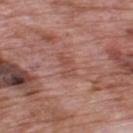Imaged during a routine full-body skin examination; the lesion was not biopsied and no histopathology is available. An algorithmic analysis of the crop reported border irregularity of about 5 on a 0–10 scale, a within-lesion color-variation index near 2/10, and a peripheral color-asymmetry measure near 0.5. Measured at roughly 3.5 mm in maximum diameter. A roughly 15 mm field-of-view crop from a total-body skin photograph. Captured under white-light illumination. A male patient, aged approximately 70. The lesion is on the upper back.